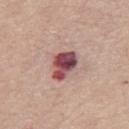Clinical impression: No biopsy was performed on this lesion — it was imaged during a full skin examination and was not determined to be concerning. Context: An algorithmic analysis of the crop reported a lesion area of about 8.5 mm², a shape eccentricity near 0.7, and a shape-asymmetry score of about 0.3 (0 = symmetric). The analysis additionally found a border-irregularity rating of about 2.5/10 and peripheral color asymmetry of about 3. It also reported an automated nevus-likeness rating near 5 out of 100 and lesion-presence confidence of about 100/100. Imaged with white-light lighting. The lesion is on the chest. A lesion tile, about 15 mm wide, cut from a 3D total-body photograph. A female subject about 70 years old.